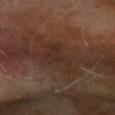No biopsy was performed on this lesion — it was imaged during a full skin examination and was not determined to be concerning. A lesion tile, about 15 mm wide, cut from a 3D total-body photograph. The patient is a male aged approximately 70. On the left forearm.